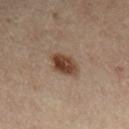  biopsy_status: not biopsied; imaged during a skin examination
  lesion_size:
    long_diameter_mm_approx: 3.5
  site: left leg
  image:
    source: total-body photography crop
    field_of_view_mm: 15
  patient:
    sex: female
    age_approx: 55
  automated_metrics:
    border_irregularity_0_10: 2.0
    nevus_likeness_0_100: 100
    lesion_detection_confidence_0_100: 100
  lighting: cross-polarized